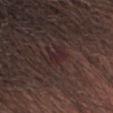A male patient, aged 63 to 67.
A lesion tile, about 15 mm wide, cut from a 3D total-body photograph.
About 3 mm across.
The total-body-photography lesion software estimated radial color variation of about 1.5. The software also gave a nevus-likeness score of about 0/100.
Located on the head or neck.